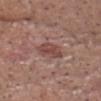Case summary:
– follow-up — catalogued during a skin exam; not biopsied
– site — the head or neck
– TBP lesion metrics — a footprint of about 6 mm² and two-axis asymmetry of about 0.25; a border-irregularity rating of about 3/10, a color-variation rating of about 2.5/10, and radial color variation of about 1; an automated nevus-likeness rating near 0 out of 100 and a lesion-detection confidence of about 100/100
– acquisition — ~15 mm crop, total-body skin-cancer survey
– size — ~4 mm (longest diameter)
– patient — male, aged approximately 65
– tile lighting — white-light illumination